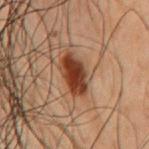anatomic site: the mid back; tile lighting: cross-polarized; image source: ~15 mm tile from a whole-body skin photo; patient: male, in their 50s; size: ≈4.5 mm.